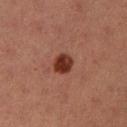<lesion>
<biopsy_status>not biopsied; imaged during a skin examination</biopsy_status>
<image>
  <source>total-body photography crop</source>
  <field_of_view_mm>15</field_of_view_mm>
</image>
<lesion_size>
  <long_diameter_mm_approx>2.5</long_diameter_mm_approx>
</lesion_size>
<patient>
  <sex>female</sex>
  <age_approx>40</age_approx>
</patient>
<lighting>cross-polarized</lighting>
<site>left lower leg</site>
</lesion>